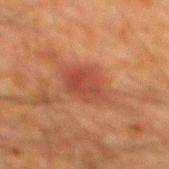follow-up: catalogued during a skin exam; not biopsied | image source: total-body-photography crop, ~15 mm field of view | size: ≈4 mm | site: the mid back | subject: male, roughly 60 years of age | image-analysis metrics: a footprint of about 11 mm², an outline eccentricity of about 0.65 (0 = round, 1 = elongated), and a shape-asymmetry score of about 0.15 (0 = symmetric); internal color variation of about 4.5 on a 0–10 scale and radial color variation of about 1.5.